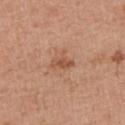Assessment:
The lesion was tiled from a total-body skin photograph and was not biopsied.